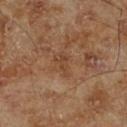acquisition — 15 mm crop, total-body photography
illumination — cross-polarized illumination
subject — male, in their 70s
body site — the right lower leg
TBP lesion metrics — an average lesion color of about L≈41 a*≈20 b*≈31 (CIELAB) and a normalized lesion–skin contrast near 5.5; a within-lesion color-variation index near 0/10